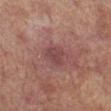Assessment: Recorded during total-body skin imaging; not selected for excision or biopsy. Clinical summary: A 15 mm close-up extracted from a 3D total-body photography capture. From the right lower leg. About 3 mm across. A male subject, approximately 65 years of age. An algorithmic analysis of the crop reported a border-irregularity index near 2.5/10 and a color-variation rating of about 2/10.